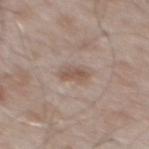Q: How was this image acquired?
A: total-body-photography crop, ~15 mm field of view
Q: Who is the patient?
A: male, aged 53 to 57
Q: Where on the body is the lesion?
A: the mid back
Q: What lighting was used for the tile?
A: white-light illumination